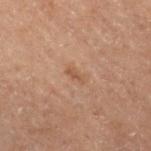The subject is a male aged 68 to 72. A 15 mm crop from a total-body photograph taken for skin-cancer surveillance. Captured under cross-polarized illumination. The lesion-visualizer software estimated a lesion area of about 2.5 mm², a shape eccentricity near 0.95, and a symmetry-axis asymmetry near 0.4. The software also gave an average lesion color of about L≈40 a*≈16 b*≈25 (CIELAB) and a lesion-to-skin contrast of about 5.5 (normalized; higher = more distinct). The software also gave a lesion-detection confidence of about 100/100. Located on the right thigh. Longest diameter approximately 2.5 mm.